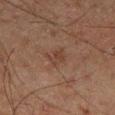This lesion was catalogued during total-body skin photography and was not selected for biopsy.
The lesion is on the left leg.
A 15 mm close-up extracted from a 3D total-body photography capture.
A male patient, approximately 60 years of age.